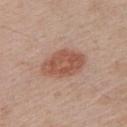Notes:
• notes — imaged on a skin check; not biopsied
• patient — male, aged around 65
• site — the chest
• image source — 15 mm crop, total-body photography
• illumination — white-light illumination